On the left upper arm. The subject is a female aged 48–52. A 15 mm crop from a total-body photograph taken for skin-cancer surveillance:
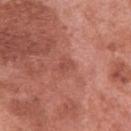histopathology: a melanoma in situ, lentigo maligna type (malignant)